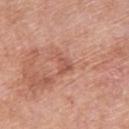follow-up = catalogued during a skin exam; not biopsied
size = ~2.5 mm (longest diameter)
patient = female, aged 58–62
imaging modality = total-body-photography crop, ~15 mm field of view
anatomic site = the upper back